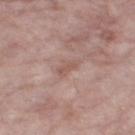Impression:
This lesion was catalogued during total-body skin photography and was not selected for biopsy.
Context:
Approximately 3 mm at its widest. A female patient approximately 70 years of age. The tile uses white-light illumination. Cropped from a total-body skin-imaging series; the visible field is about 15 mm. Automated image analysis of the tile measured a lesion color around L≈55 a*≈20 b*≈24 in CIELAB and a normalized lesion–skin contrast near 5.5. The software also gave a border-irregularity rating of about 3.5/10 and peripheral color asymmetry of about 0. It also reported an automated nevus-likeness rating near 0 out of 100 and a detector confidence of about 100 out of 100 that the crop contains a lesion. On the right thigh.